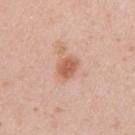<lesion>
  <biopsy_status>not biopsied; imaged during a skin examination</biopsy_status>
  <patient>
    <sex>female</sex>
    <age_approx>30</age_approx>
  </patient>
  <image>
    <source>total-body photography crop</source>
    <field_of_view_mm>15</field_of_view_mm>
  </image>
  <automated_metrics>
    <cielab_L>60</cielab_L>
    <cielab_a>24</cielab_a>
    <cielab_b>32</cielab_b>
    <vs_skin_darker_L>11.0</vs_skin_darker_L>
    <vs_skin_contrast_norm>8.0</vs_skin_contrast_norm>
    <color_variation_0_10>3.0</color_variation_0_10>
    <peripheral_color_asymmetry>1.0</peripheral_color_asymmetry>
  </automated_metrics>
  <lighting>white-light</lighting>
  <site>right upper arm</site>
</lesion>